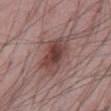{
  "biopsy_status": "not biopsied; imaged during a skin examination",
  "patient": {
    "sex": "male",
    "age_approx": 65
  },
  "lighting": "white-light",
  "lesion_size": {
    "long_diameter_mm_approx": 5.5
  },
  "site": "abdomen",
  "automated_metrics": {
    "eccentricity": 0.8,
    "shape_asymmetry": 0.25,
    "color_variation_0_10": 6.0,
    "peripheral_color_asymmetry": 1.5
  },
  "image": {
    "source": "total-body photography crop",
    "field_of_view_mm": 15
  }
}